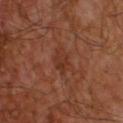Q: What is the lesion's diameter?
A: ~2.5 mm (longest diameter)
Q: Where on the body is the lesion?
A: the back
Q: What lighting was used for the tile?
A: cross-polarized
Q: What is the imaging modality?
A: 15 mm crop, total-body photography
Q: Who is the patient?
A: male, in their mid- to late 50s